A close-up tile cropped from a whole-body skin photograph, about 15 mm across. A male patient, roughly 75 years of age. Automated image analysis of the tile measured an area of roughly 30 mm², an eccentricity of roughly 0.85, and two-axis asymmetry of about 0.4. This is a white-light tile. The lesion is located on the mid back. Approximately 9.5 mm at its widest: 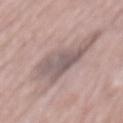Q: What is the histopathologic diagnosis?
A: an invasive melanoma, superficial spreading type; Breslow depth 0.3 mm, mitotic rate <1/mm²